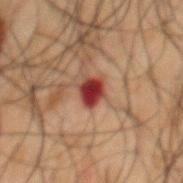No biopsy was performed on this lesion — it was imaged during a full skin examination and was not determined to be concerning.
Automated tile analysis of the lesion measured a mean CIELAB color near L≈28 a*≈27 b*≈22, a lesion–skin lightness drop of about 16, and a lesion-to-skin contrast of about 14.5 (normalized; higher = more distinct). The software also gave border irregularity of about 2 on a 0–10 scale and peripheral color asymmetry of about 2. And it measured a lesion-detection confidence of about 100/100.
A male patient, in their 50s.
The lesion is on the back.
About 2.5 mm across.
Cropped from a whole-body photographic skin survey; the tile spans about 15 mm.